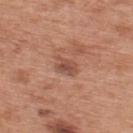Context: Captured under white-light illumination. Cropped from a total-body skin-imaging series; the visible field is about 15 mm. Approximately 3 mm at its widest. A male patient about 70 years old. The lesion is on the upper back.